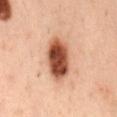{"biopsy_status": "not biopsied; imaged during a skin examination", "automated_metrics": {"eccentricity": 0.85}, "site": "mid back", "lighting": "cross-polarized", "image": {"source": "total-body photography crop", "field_of_view_mm": 15}, "lesion_size": {"long_diameter_mm_approx": 6.0}, "patient": {"sex": "male", "age_approx": 55}}